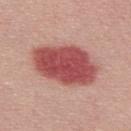The total-body-photography lesion software estimated a footprint of about 28 mm², a shape eccentricity near 0.8, and a shape-asymmetry score of about 0.15 (0 = symmetric). It also reported a lesion color around L≈51 a*≈32 b*≈24 in CIELAB, about 17 CIELAB-L* units darker than the surrounding skin, and a normalized lesion–skin contrast near 11. And it measured a border-irregularity rating of about 1.5/10, internal color variation of about 4 on a 0–10 scale, and peripheral color asymmetry of about 1.
Measured at roughly 7.5 mm in maximum diameter.
A male subject aged approximately 30.
This image is a 15 mm lesion crop taken from a total-body photograph.
Captured under white-light illumination.